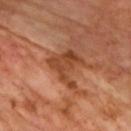Impression:
The lesion was photographed on a routine skin check and not biopsied; there is no pathology result.
Background:
A male patient in their mid- to late 60s. A close-up tile cropped from a whole-body skin photograph, about 15 mm across. Captured under cross-polarized illumination. Automated tile analysis of the lesion measured a lesion area of about 10 mm², an eccentricity of roughly 0.55, and a symmetry-axis asymmetry near 0.6. And it measured border irregularity of about 7 on a 0–10 scale, a within-lesion color-variation index near 3.5/10, and peripheral color asymmetry of about 1.5. The analysis additionally found an automated nevus-likeness rating near 0 out of 100 and lesion-presence confidence of about 100/100. About 5 mm across.